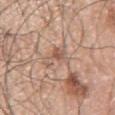Assessment:
The lesion was photographed on a routine skin check and not biopsied; there is no pathology result.
Background:
Automated image analysis of the tile measured a lesion area of about 5 mm², an eccentricity of roughly 0.75, and a shape-asymmetry score of about 0.5 (0 = symmetric). The software also gave a color-variation rating of about 3.5/10. And it measured a nevus-likeness score of about 0/100 and a detector confidence of about 100 out of 100 that the crop contains a lesion. Longest diameter approximately 3.5 mm. A male subject, roughly 70 years of age. A 15 mm crop from a total-body photograph taken for skin-cancer surveillance. The lesion is located on the left arm.